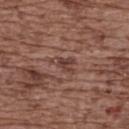Captured under white-light illumination.
The recorded lesion diameter is about 3 mm.
The total-body-photography lesion software estimated a shape eccentricity near 0.8 and a symmetry-axis asymmetry near 0.5. The analysis additionally found border irregularity of about 5 on a 0–10 scale, a color-variation rating of about 2.5/10, and peripheral color asymmetry of about 1. And it measured a lesion-detection confidence of about 80/100.
The patient is a female aged around 75.
On the upper back.
A 15 mm crop from a total-body photograph taken for skin-cancer surveillance.